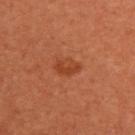| feature | finding |
|---|---|
| biopsy status | no biopsy performed (imaged during a skin exam) |
| TBP lesion metrics | an eccentricity of roughly 0.8 and a symmetry-axis asymmetry near 0.25; a classifier nevus-likeness of about 85/100 and a detector confidence of about 100 out of 100 that the crop contains a lesion |
| image | ~15 mm tile from a whole-body skin photo |
| patient | female, aged approximately 50 |
| lighting | cross-polarized illumination |
| size | ~3 mm (longest diameter) |